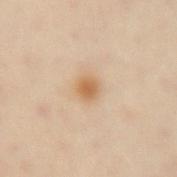biopsy status = no biopsy performed (imaged during a skin exam); lighting = cross-polarized; image = ~15 mm tile from a whole-body skin photo; location = the arm; subject = male, about 55 years old.